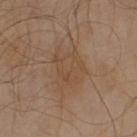Notes:
• follow-up · imaged on a skin check; not biopsied
• patient · male, aged around 65
• body site · the arm
• automated lesion analysis · a nevus-likeness score of about 0/100 and lesion-presence confidence of about 100/100
• image · ~15 mm tile from a whole-body skin photo
• size · ~5 mm (longest diameter)
• lighting · cross-polarized